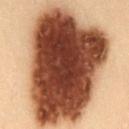Assessment: The lesion was photographed on a routine skin check and not biopsied; there is no pathology result. Background: This is a cross-polarized tile. A male patient, aged 53–57. A 15 mm close-up tile from a total-body photography series done for melanoma screening. The lesion's longest dimension is about 13.5 mm. The lesion is on the abdomen. Automated image analysis of the tile measured a lesion-detection confidence of about 100/100.